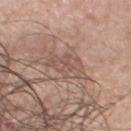Imaged during a routine full-body skin examination; the lesion was not biopsied and no histopathology is available. Located on the chest. A 15 mm close-up extracted from a 3D total-body photography capture. A male subject, approximately 70 years of age. Measured at roughly 5.5 mm in maximum diameter.